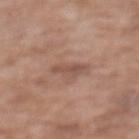Impression:
The lesion was tiled from a total-body skin photograph and was not biopsied.